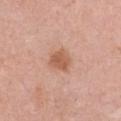biopsy_status: not biopsied; imaged during a skin examination
image:
  source: total-body photography crop
  field_of_view_mm: 15
patient:
  sex: female
  age_approx: 40
lighting: white-light
automated_metrics:
  area_mm2_approx: 5.5
  eccentricity: 0.35
  shape_asymmetry: 0.2
  border_irregularity_0_10: 2.0
  color_variation_0_10: 2.5
  nevus_likeness_0_100: 85
  lesion_detection_confidence_0_100: 100
site: chest
lesion_size:
  long_diameter_mm_approx: 2.5